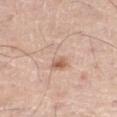This lesion was catalogued during total-body skin photography and was not selected for biopsy. The lesion is located on the leg. A lesion tile, about 15 mm wide, cut from a 3D total-body photograph. Longest diameter approximately 4.5 mm. The patient is a male aged 78–82. Captured under white-light illumination.